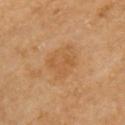Q: Was this lesion biopsied?
A: catalogued during a skin exam; not biopsied